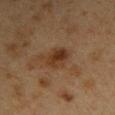Part of a total-body skin-imaging series; this lesion was reviewed on a skin check and was not flagged for biopsy.
Imaged with cross-polarized lighting.
From the back.
Longest diameter approximately 3 mm.
A region of skin cropped from a whole-body photographic capture, roughly 15 mm wide.
The subject is a female roughly 40 years of age.